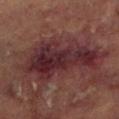The lesion was tiled from a total-body skin photograph and was not biopsied. The lesion-visualizer software estimated an area of roughly 29 mm² and a shape eccentricity near 0.85. The software also gave a nevus-likeness score of about 0/100 and a lesion-detection confidence of about 95/100. The lesion is located on the left lower leg. A close-up tile cropped from a whole-body skin photograph, about 15 mm across. Imaged with cross-polarized lighting. A male subject aged approximately 70.